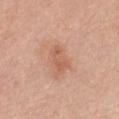Context: This is a white-light tile. The lesion-visualizer software estimated a lesion area of about 5 mm², an eccentricity of roughly 0.85, and two-axis asymmetry of about 0.4. It also reported an average lesion color of about L≈59 a*≈23 b*≈32 (CIELAB), about 8 CIELAB-L* units darker than the surrounding skin, and a lesion-to-skin contrast of about 5.5 (normalized; higher = more distinct). The analysis additionally found border irregularity of about 4.5 on a 0–10 scale. Located on the chest. Longest diameter approximately 3.5 mm. A female subject, aged around 40. A region of skin cropped from a whole-body photographic capture, roughly 15 mm wide.